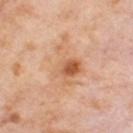workup=imaged on a skin check; not biopsied
lighting=cross-polarized illumination
subject=female, aged around 55
lesion diameter=about 7 mm
TBP lesion metrics=an area of roughly 14 mm², a shape eccentricity near 0.85, and two-axis asymmetry of about 0.45; a mean CIELAB color near L≈63 a*≈22 b*≈36 and a normalized border contrast of about 6.5; a color-variation rating of about 8.5/10 and a peripheral color-asymmetry measure near 2.5; a nevus-likeness score of about 55/100 and a detector confidence of about 100 out of 100 that the crop contains a lesion
anatomic site=the right thigh
image source=~15 mm tile from a whole-body skin photo